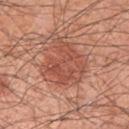<case>
<biopsy_status>not biopsied; imaged during a skin examination</biopsy_status>
<site>left upper arm</site>
<patient>
  <sex>male</sex>
  <age_approx>60</age_approx>
</patient>
<image>
  <source>total-body photography crop</source>
  <field_of_view_mm>15</field_of_view_mm>
</image>
</case>